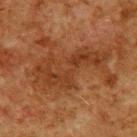Recorded during total-body skin imaging; not selected for excision or biopsy.
The lesion is located on the upper back.
The lesion-visualizer software estimated a lesion area of about 17 mm² and two-axis asymmetry of about 0.6. The analysis additionally found a color-variation rating of about 3/10 and radial color variation of about 1.
A region of skin cropped from a whole-body photographic capture, roughly 15 mm wide.
Captured under cross-polarized illumination.
Longest diameter approximately 8.5 mm.
A male subject roughly 80 years of age.